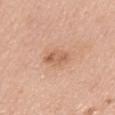Recorded during total-body skin imaging; not selected for excision or biopsy.
The lesion is on the mid back.
The patient is a female about 60 years old.
Imaged with white-light lighting.
A 15 mm close-up tile from a total-body photography series done for melanoma screening.
The total-body-photography lesion software estimated a lesion area of about 5 mm² and an outline eccentricity of about 0.85 (0 = round, 1 = elongated). And it measured about 9 CIELAB-L* units darker than the surrounding skin and a normalized border contrast of about 6.5. It also reported internal color variation of about 4 on a 0–10 scale and a peripheral color-asymmetry measure near 1. The analysis additionally found a classifier nevus-likeness of about 5/100 and a lesion-detection confidence of about 100/100.